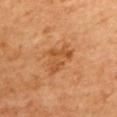biopsy status=no biopsy performed (imaged during a skin exam); image source=total-body-photography crop, ~15 mm field of view; subject=female; site=the upper back.